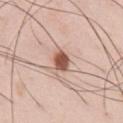Impression: Imaged during a routine full-body skin examination; the lesion was not biopsied and no histopathology is available. Acquisition and patient details: From the chest. The tile uses white-light illumination. A male patient in their mid-30s. Cropped from a total-body skin-imaging series; the visible field is about 15 mm.